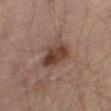No biopsy was performed on this lesion — it was imaged during a full skin examination and was not determined to be concerning. Cropped from a total-body skin-imaging series; the visible field is about 15 mm. Captured under cross-polarized illumination. Approximately 4.5 mm at its widest. Automated tile analysis of the lesion measured a classifier nevus-likeness of about 70/100. From the right thigh. The patient is a male aged 58 to 62.